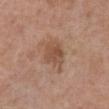No biopsy was performed on this lesion — it was imaged during a full skin examination and was not determined to be concerning. The total-body-photography lesion software estimated an average lesion color of about L≈51 a*≈20 b*≈30 (CIELAB), a lesion–skin lightness drop of about 9, and a normalized lesion–skin contrast near 6.5. A region of skin cropped from a whole-body photographic capture, roughly 15 mm wide. Approximately 4.5 mm at its widest. The lesion is located on the abdomen. A male patient approximately 70 years of age. Captured under white-light illumination.